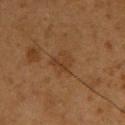The lesion was photographed on a routine skin check and not biopsied; there is no pathology result.
The recorded lesion diameter is about 3 mm.
A male patient, in their mid- to late 50s.
A 15 mm close-up extracted from a 3D total-body photography capture.
Automated image analysis of the tile measured an area of roughly 6.5 mm², an outline eccentricity of about 0.2 (0 = round, 1 = elongated), and a symmetry-axis asymmetry near 0.25. And it measured about 5 CIELAB-L* units darker than the surrounding skin and a normalized lesion–skin contrast near 5. It also reported a border-irregularity rating of about 2.5/10, a within-lesion color-variation index near 3/10, and a peripheral color-asymmetry measure near 1. The software also gave a classifier nevus-likeness of about 0/100 and a lesion-detection confidence of about 100/100.
Imaged with cross-polarized lighting.
From the upper back.